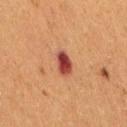Imaged during a routine full-body skin examination; the lesion was not biopsied and no histopathology is available. This image is a 15 mm lesion crop taken from a total-body photograph. A female patient approximately 50 years of age. On the right thigh.